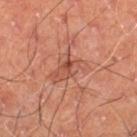  site: left thigh
  patient:
    sex: male
    age_approx: 60
  image:
    source: total-body photography crop
    field_of_view_mm: 15